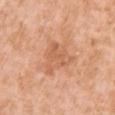Clinical impression:
The lesion was photographed on a routine skin check and not biopsied; there is no pathology result.
Background:
The recorded lesion diameter is about 4.5 mm. Cropped from a total-body skin-imaging series; the visible field is about 15 mm. On the right upper arm. A female subject in their 40s. The tile uses white-light illumination.